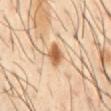Context:
A male subject, roughly 40 years of age. From the chest. About 3 mm across. A 15 mm close-up extracted from a 3D total-body photography capture. The tile uses cross-polarized illumination.